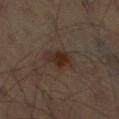This lesion was catalogued during total-body skin photography and was not selected for biopsy.
A 15 mm close-up extracted from a 3D total-body photography capture.
The lesion is on the right thigh.
A male patient, aged approximately 65.
Measured at roughly 4 mm in maximum diameter.
The total-body-photography lesion software estimated an average lesion color of about L≈20 a*≈12 b*≈18 (CIELAB), about 6 CIELAB-L* units darker than the surrounding skin, and a lesion-to-skin contrast of about 8.5 (normalized; higher = more distinct). It also reported a border-irregularity index near 2.5/10, a color-variation rating of about 3/10, and radial color variation of about 0.5.